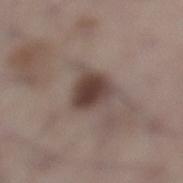Clinical impression:
This lesion was catalogued during total-body skin photography and was not selected for biopsy.
Image and clinical context:
Imaged with white-light lighting. The total-body-photography lesion software estimated a border-irregularity rating of about 3/10, a color-variation rating of about 4/10, and peripheral color asymmetry of about 1. The patient is a male aged 68 to 72. A lesion tile, about 15 mm wide, cut from a 3D total-body photograph. The lesion is located on the left lower leg.